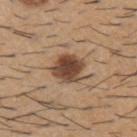This lesion was catalogued during total-body skin photography and was not selected for biopsy. Approximately 4.5 mm at its widest. A lesion tile, about 15 mm wide, cut from a 3D total-body photograph. This is a white-light tile. The lesion is on the upper back. A male subject approximately 60 years of age.